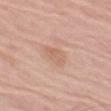Notes:
– notes: catalogued during a skin exam; not biopsied
– location: the leg
– illumination: white-light illumination
– patient: male, in their 80s
– imaging modality: 15 mm crop, total-body photography
– lesion diameter: ≈3.5 mm
– image-analysis metrics: an area of roughly 5.5 mm²; an average lesion color of about L≈63 a*≈20 b*≈30 (CIELAB), about 6 CIELAB-L* units darker than the surrounding skin, and a normalized border contrast of about 5; a border-irregularity index near 2/10, a color-variation rating of about 2/10, and peripheral color asymmetry of about 0.5